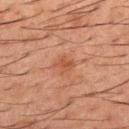Q: Is there a histopathology result?
A: imaged on a skin check; not biopsied
Q: What is the lesion's diameter?
A: about 2.5 mm
Q: Where on the body is the lesion?
A: the mid back
Q: What lighting was used for the tile?
A: cross-polarized
Q: Automated lesion metrics?
A: a lesion color around L≈40 a*≈21 b*≈28 in CIELAB, a lesion–skin lightness drop of about 6, and a normalized border contrast of about 6; a border-irregularity rating of about 2.5/10, a within-lesion color-variation index near 1/10, and radial color variation of about 0.5; a classifier nevus-likeness of about 0/100 and a detector confidence of about 100 out of 100 that the crop contains a lesion
Q: What kind of image is this?
A: ~15 mm crop, total-body skin-cancer survey
Q: What are the patient's age and sex?
A: male, in their 50s